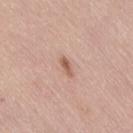<record>
  <biopsy_status>not biopsied; imaged during a skin examination</biopsy_status>
  <site>right thigh</site>
  <image>
    <source>total-body photography crop</source>
    <field_of_view_mm>15</field_of_view_mm>
  </image>
  <patient>
    <sex>female</sex>
    <age_approx>65</age_approx>
  </patient>
</record>